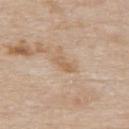Clinical impression: Captured during whole-body skin photography for melanoma surveillance; the lesion was not biopsied. Acquisition and patient details: Automated image analysis of the tile measured a nevus-likeness score of about 0/100 and a detector confidence of about 100 out of 100 that the crop contains a lesion. About 3 mm across. The subject is a male in their mid-80s or older. This is a white-light tile. A roughly 15 mm field-of-view crop from a total-body skin photograph. On the upper back.